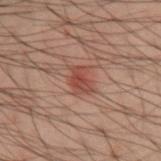follow-up=catalogued during a skin exam; not biopsied | location=the right forearm | patient=male, about 40 years old | image source=~15 mm tile from a whole-body skin photo.